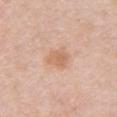  biopsy_status: not biopsied; imaged during a skin examination
  patient:
    sex: female
    age_approx: 50
  image:
    source: total-body photography crop
    field_of_view_mm: 15
  site: right upper arm
  lighting: white-light